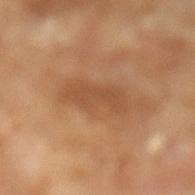This lesion was catalogued during total-body skin photography and was not selected for biopsy. Longest diameter approximately 5 mm. A 15 mm crop from a total-body photograph taken for skin-cancer surveillance. The patient is a male aged around 65. The tile uses cross-polarized illumination.